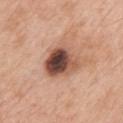| feature | finding |
|---|---|
| follow-up | catalogued during a skin exam; not biopsied |
| image source | total-body-photography crop, ~15 mm field of view |
| TBP lesion metrics | a border-irregularity index near 2.5/10 and internal color variation of about 10 on a 0–10 scale; a classifier nevus-likeness of about 20/100 and a detector confidence of about 100 out of 100 that the crop contains a lesion |
| patient | male, approximately 70 years of age |
| location | the back |